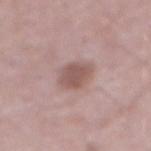Assessment: No biopsy was performed on this lesion — it was imaged during a full skin examination and was not determined to be concerning. Background: A 15 mm crop from a total-body photograph taken for skin-cancer surveillance. A male subject in their mid-70s. From the abdomen. The tile uses white-light illumination. Measured at roughly 3.5 mm in maximum diameter.